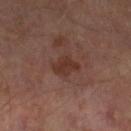Context:
Imaged with cross-polarized lighting. The lesion-visualizer software estimated an area of roughly 6.5 mm² and an eccentricity of roughly 0.8. The analysis additionally found a lesion color around L≈32 a*≈19 b*≈23 in CIELAB and roughly 7 lightness units darker than nearby skin. The analysis additionally found border irregularity of about 3.5 on a 0–10 scale, internal color variation of about 2 on a 0–10 scale, and peripheral color asymmetry of about 0.5. A region of skin cropped from a whole-body photographic capture, roughly 15 mm wide. The lesion is located on the leg. A male subject, approximately 65 years of age. The lesion's longest dimension is about 3.5 mm.